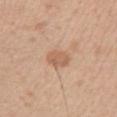| field | value |
|---|---|
| workup | imaged on a skin check; not biopsied |
| subject | male, aged around 45 |
| site | the right upper arm |
| imaging modality | ~15 mm tile from a whole-body skin photo |